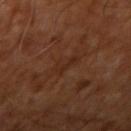This lesion was catalogued during total-body skin photography and was not selected for biopsy.
The lesion is on the arm.
This image is a 15 mm lesion crop taken from a total-body photograph.
A patient aged approximately 65.